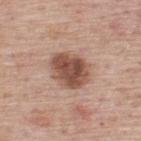subject: male, roughly 75 years of age
location: the upper back
acquisition: 15 mm crop, total-body photography